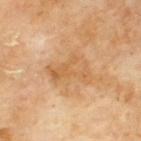Clinical impression: No biopsy was performed on this lesion — it was imaged during a full skin examination and was not determined to be concerning. Clinical summary: A 15 mm crop from a total-body photograph taken for skin-cancer surveillance. On the upper back. A male subject aged around 70.